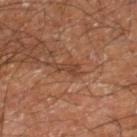Impression: This lesion was catalogued during total-body skin photography and was not selected for biopsy. Acquisition and patient details: About 3 mm across. A close-up tile cropped from a whole-body skin photograph, about 15 mm across. A male patient, aged approximately 60. The lesion is located on the right lower leg. The total-body-photography lesion software estimated a border-irregularity index near 5.5/10 and radial color variation of about 0.5. It also reported a classifier nevus-likeness of about 0/100 and a lesion-detection confidence of about 100/100.